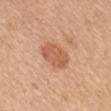  patient:
    sex: female
    age_approx: 55
  automated_metrics:
    area_mm2_approx: 9.0
    eccentricity: 0.8
    shape_asymmetry: 0.2
    vs_skin_contrast_norm: 6.5
  image:
    source: total-body photography crop
    field_of_view_mm: 15
  lighting: white-light
  lesion_size:
    long_diameter_mm_approx: 4.0
  site: mid back